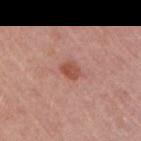workup: no biopsy performed (imaged during a skin exam)
image source: ~15 mm tile from a whole-body skin photo
subject: male, in their mid-50s
body site: the arm